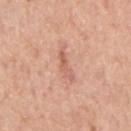{"biopsy_status": "not biopsied; imaged during a skin examination", "lesion_size": {"long_diameter_mm_approx": 4.0}, "image": {"source": "total-body photography crop", "field_of_view_mm": 15}, "patient": {"sex": "male", "age_approx": 75}, "lighting": "white-light", "site": "right upper arm"}